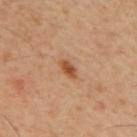Impression:
Imaged during a routine full-body skin examination; the lesion was not biopsied and no histopathology is available.
Image and clinical context:
The tile uses cross-polarized illumination. The lesion is located on the mid back. A male patient aged approximately 60. The lesion's longest dimension is about 2.5 mm. A lesion tile, about 15 mm wide, cut from a 3D total-body photograph.